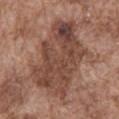* biopsy status — no biopsy performed (imaged during a skin exam)
* image — total-body-photography crop, ~15 mm field of view
* patient — male, aged around 75
* image-analysis metrics — an average lesion color of about L≈45 a*≈20 b*≈26 (CIELAB) and a normalized border contrast of about 8.5; an automated nevus-likeness rating near 0 out of 100 and lesion-presence confidence of about 95/100
* lesion size — ≈9.5 mm
* illumination — white-light
* site — the abdomen